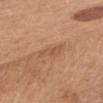{"automated_metrics": {"area_mm2_approx": 4.0, "vs_skin_darker_L": 7.0, "vs_skin_contrast_norm": 4.5, "border_irregularity_0_10": 3.5, "color_variation_0_10": 1.0, "nevus_likeness_0_100": 0, "lesion_detection_confidence_0_100": 100}, "patient": {"sex": "female", "age_approx": 55}, "site": "chest", "lesion_size": {"long_diameter_mm_approx": 3.5}, "image": {"source": "total-body photography crop", "field_of_view_mm": 15}}